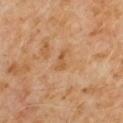Q: Was this lesion biopsied?
A: catalogued during a skin exam; not biopsied
Q: How large is the lesion?
A: about 3 mm
Q: How was the tile lit?
A: cross-polarized illumination
Q: Lesion location?
A: the arm
Q: Automated lesion metrics?
A: a shape eccentricity near 0.9 and a symmetry-axis asymmetry near 0.4; an average lesion color of about L≈51 a*≈20 b*≈37 (CIELAB) and about 7 CIELAB-L* units darker than the surrounding skin; a color-variation rating of about 0.5/10; a detector confidence of about 100 out of 100 that the crop contains a lesion
Q: Patient demographics?
A: male, about 60 years old
Q: What is the imaging modality?
A: ~15 mm crop, total-body skin-cancer survey